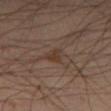The lesion was tiled from a total-body skin photograph and was not biopsied.
The lesion is on the left thigh.
A male subject, aged 43 to 47.
A 15 mm crop from a total-body photograph taken for skin-cancer surveillance.